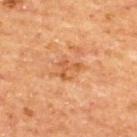Q: Was a biopsy performed?
A: total-body-photography surveillance lesion; no biopsy